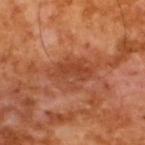Q: Was this lesion biopsied?
A: total-body-photography surveillance lesion; no biopsy
Q: What are the patient's age and sex?
A: male, approximately 65 years of age
Q: How was this image acquired?
A: total-body-photography crop, ~15 mm field of view
Q: Automated lesion metrics?
A: a lesion area of about 11 mm² and a shape eccentricity near 0.8; a border-irregularity rating of about 3.5/10, a color-variation rating of about 4.5/10, and a peripheral color-asymmetry measure near 1.5; a nevus-likeness score of about 0/100 and a lesion-detection confidence of about 100/100
Q: Lesion size?
A: about 5 mm
Q: What lighting was used for the tile?
A: cross-polarized